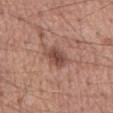* notes — no biopsy performed (imaged during a skin exam)